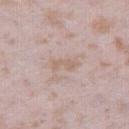Recorded during total-body skin imaging; not selected for excision or biopsy. Imaged with white-light lighting. The subject is a female aged 23 to 27. Longest diameter approximately 3 mm. A 15 mm close-up tile from a total-body photography series done for melanoma screening. Located on the right thigh.